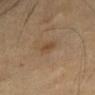Recorded during total-body skin imaging; not selected for excision or biopsy. This is a cross-polarized tile. A 15 mm close-up tile from a total-body photography series done for melanoma screening. The lesion is on the abdomen. The lesion-visualizer software estimated a mean CIELAB color near L≈40 a*≈14 b*≈27, roughly 6 lightness units darker than nearby skin, and a lesion-to-skin contrast of about 5.5 (normalized; higher = more distinct). It also reported a peripheral color-asymmetry measure near 1. The lesion's longest dimension is about 2.5 mm. A female patient, in their mid- to late 50s.